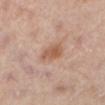The lesion was tiled from a total-body skin photograph and was not biopsied. The lesion-visualizer software estimated a lesion–skin lightness drop of about 9 and a normalized lesion–skin contrast near 7. And it measured a color-variation rating of about 3/10 and peripheral color asymmetry of about 1. It also reported a classifier nevus-likeness of about 80/100 and lesion-presence confidence of about 100/100. Measured at roughly 3 mm in maximum diameter. The lesion is on the right leg. A female patient, in their 40s. A 15 mm crop from a total-body photograph taken for skin-cancer surveillance. The tile uses cross-polarized illumination.